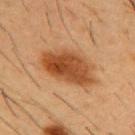Impression: Recorded during total-body skin imaging; not selected for excision or biopsy. Background: Measured at roughly 6.5 mm in maximum diameter. A roughly 15 mm field-of-view crop from a total-body skin photograph. The subject is a male aged 53–57. On the chest. Automated tile analysis of the lesion measured a lesion area of about 16 mm², a shape eccentricity near 0.85, and two-axis asymmetry of about 0.25. It also reported a border-irregularity rating of about 2.5/10, a within-lesion color-variation index near 4.5/10, and a peripheral color-asymmetry measure near 1.5. The software also gave a nevus-likeness score of about 100/100 and a detector confidence of about 100 out of 100 that the crop contains a lesion.